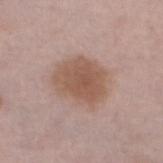Impression: Part of a total-body skin-imaging series; this lesion was reviewed on a skin check and was not flagged for biopsy. Background: A region of skin cropped from a whole-body photographic capture, roughly 15 mm wide. Approximately 5.5 mm at its widest. Captured under white-light illumination. A male subject, roughly 65 years of age. On the leg.